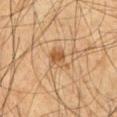Findings:
– follow-up: catalogued during a skin exam; not biopsied
– image source: ~15 mm tile from a whole-body skin photo
– body site: the front of the torso
– size: ≈3 mm
– subject: male, in their mid- to late 60s
– lighting: cross-polarized illumination
– automated lesion analysis: an outline eccentricity of about 0.65 (0 = round, 1 = elongated) and a symmetry-axis asymmetry near 0.35; a lesion color around L≈46 a*≈17 b*≈33 in CIELAB and roughly 8 lightness units darker than nearby skin; lesion-presence confidence of about 100/100